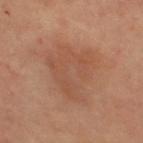Imaged during a routine full-body skin examination; the lesion was not biopsied and no histopathology is available. Automated image analysis of the tile measured a mean CIELAB color near L≈52 a*≈21 b*≈30 and a lesion–skin lightness drop of about 6. It also reported a border-irregularity index near 5.5/10, internal color variation of about 4 on a 0–10 scale, and radial color variation of about 1.5. The analysis additionally found a classifier nevus-likeness of about 0/100 and lesion-presence confidence of about 100/100. On the upper back. A male subject in their mid-50s. A region of skin cropped from a whole-body photographic capture, roughly 15 mm wide.